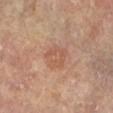The lesion is on the leg.
A lesion tile, about 15 mm wide, cut from a 3D total-body photograph.
About 3.5 mm across.
The total-body-photography lesion software estimated a lesion area of about 7 mm², an outline eccentricity of about 0.45 (0 = round, 1 = elongated), and a symmetry-axis asymmetry near 0.3. It also reported an average lesion color of about L≈56 a*≈22 b*≈31 (CIELAB) and a lesion-to-skin contrast of about 4.5 (normalized; higher = more distinct). The analysis additionally found a detector confidence of about 100 out of 100 that the crop contains a lesion.
Captured under cross-polarized illumination.
The patient is a female roughly 75 years of age.